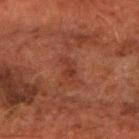A region of skin cropped from a whole-body photographic capture, roughly 15 mm wide. This is a cross-polarized tile. The lesion is on the right forearm. A male subject roughly 60 years of age. The lesion's longest dimension is about 3 mm. An algorithmic analysis of the crop reported a footprint of about 3.5 mm² and two-axis asymmetry of about 0.5. The analysis additionally found an automated nevus-likeness rating near 0 out of 100 and a detector confidence of about 100 out of 100 that the crop contains a lesion.